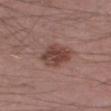Captured under white-light illumination. A male patient, in their mid-50s. A 15 mm close-up extracted from a 3D total-body photography capture. About 4 mm across. From the right thigh.